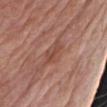Clinical impression:
Imaged during a routine full-body skin examination; the lesion was not biopsied and no histopathology is available.
Acquisition and patient details:
A male subject aged approximately 70. The lesion is on the left upper arm. The recorded lesion diameter is about 2.5 mm. A region of skin cropped from a whole-body photographic capture, roughly 15 mm wide. The lesion-visualizer software estimated a shape eccentricity near 0.85 and two-axis asymmetry of about 0.35. And it measured a lesion color around L≈47 a*≈24 b*≈28 in CIELAB, a lesion–skin lightness drop of about 7, and a normalized lesion–skin contrast near 6. The software also gave border irregularity of about 4 on a 0–10 scale and a color-variation rating of about 0/10. The tile uses white-light illumination.